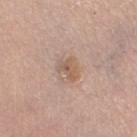No biopsy was performed on this lesion — it was imaged during a full skin examination and was not determined to be concerning.
An algorithmic analysis of the crop reported an area of roughly 4 mm², an eccentricity of roughly 0.7, and a symmetry-axis asymmetry near 0.4. And it measured a lesion color around L≈58 a*≈18 b*≈28 in CIELAB, a lesion–skin lightness drop of about 8, and a normalized lesion–skin contrast near 6.5. And it measured a border-irregularity index near 4.5/10, internal color variation of about 3 on a 0–10 scale, and radial color variation of about 1. It also reported a nevus-likeness score of about 0/100 and a detector confidence of about 100 out of 100 that the crop contains a lesion.
From the left thigh.
This image is a 15 mm lesion crop taken from a total-body photograph.
Captured under white-light illumination.
A female patient, approximately 65 years of age.